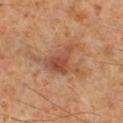{"biopsy_status": "not biopsied; imaged during a skin examination", "image": {"source": "total-body photography crop", "field_of_view_mm": 15}, "site": "right lower leg", "patient": {"sex": "male", "age_approx": 60}, "lighting": "cross-polarized"}